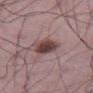Impression:
Captured during whole-body skin photography for melanoma surveillance; the lesion was not biopsied.
Background:
The subject is a male aged 48 to 52. Captured under white-light illumination. A lesion tile, about 15 mm wide, cut from a 3D total-body photograph. On the leg. Measured at roughly 3.5 mm in maximum diameter.